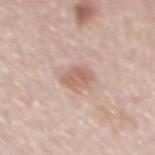{"biopsy_status": "not biopsied; imaged during a skin examination", "patient": {"sex": "male", "age_approx": 60}, "automated_metrics": {"area_mm2_approx": 5.0, "eccentricity": 0.75, "shape_asymmetry": 0.2, "vs_skin_darker_L": 10.0, "vs_skin_contrast_norm": 6.5, "border_irregularity_0_10": 2.0, "color_variation_0_10": 2.0, "peripheral_color_asymmetry": 0.5}, "lesion_size": {"long_diameter_mm_approx": 3.0}, "site": "mid back", "lighting": "white-light", "image": {"source": "total-body photography crop", "field_of_view_mm": 15}}